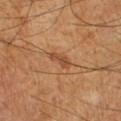follow-up = total-body-photography surveillance lesion; no biopsy | location = the left lower leg | patient = male, about 65 years old | image = 15 mm crop, total-body photography.